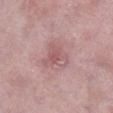The lesion is located on the right lower leg. A female subject, approximately 60 years of age. The tile uses white-light illumination. The total-body-photography lesion software estimated an area of roughly 8.5 mm², an eccentricity of roughly 0.45, and a symmetry-axis asymmetry near 0.45. It also reported an average lesion color of about L≈57 a*≈23 b*≈19 (CIELAB), roughly 8 lightness units darker than nearby skin, and a normalized border contrast of about 5.5. Approximately 3.5 mm at its widest. Cropped from a whole-body photographic skin survey; the tile spans about 15 mm.